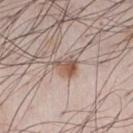Q: Was a biopsy performed?
A: total-body-photography surveillance lesion; no biopsy
Q: What is the lesion's diameter?
A: ~3 mm (longest diameter)
Q: What is the imaging modality?
A: total-body-photography crop, ~15 mm field of view
Q: What are the patient's age and sex?
A: male, aged 33–37
Q: What is the anatomic site?
A: the chest
Q: Automated lesion metrics?
A: a lesion area of about 5 mm², a shape eccentricity near 0.65, and a symmetry-axis asymmetry near 0.4; border irregularity of about 3.5 on a 0–10 scale, internal color variation of about 5.5 on a 0–10 scale, and radial color variation of about 2; an automated nevus-likeness rating near 90 out of 100 and lesion-presence confidence of about 100/100
Q: How was the tile lit?
A: white-light